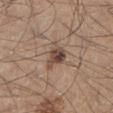workup: no biopsy performed (imaged during a skin exam) | automated lesion analysis: an area of roughly 5 mm² and two-axis asymmetry of about 0.35; a border-irregularity index near 3/10 and radial color variation of about 2.5; an automated nevus-likeness rating near 85 out of 100 and lesion-presence confidence of about 100/100 | body site: the left lower leg | subject: male, roughly 45 years of age | illumination: white-light | lesion size: about 3 mm | acquisition: 15 mm crop, total-body photography.